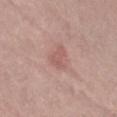Q: Is there a histopathology result?
A: no biopsy performed (imaged during a skin exam)
Q: Who is the patient?
A: female, aged 63–67
Q: Where on the body is the lesion?
A: the left thigh
Q: What is the imaging modality?
A: ~15 mm crop, total-body skin-cancer survey